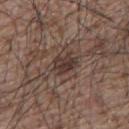| field | value |
|---|---|
| workup | no biopsy performed (imaged during a skin exam) |